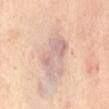  biopsy_status: not biopsied; imaged during a skin examination
  site: abdomen
  lesion_size:
    long_diameter_mm_approx: 5.0
  lighting: cross-polarized
  image:
    source: total-body photography crop
    field_of_view_mm: 15
  patient:
    sex: female
    age_approx: 60